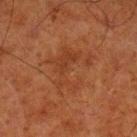<lesion>
  <image>
    <source>total-body photography crop</source>
    <field_of_view_mm>15</field_of_view_mm>
  </image>
  <site>left lower leg</site>
  <lighting>cross-polarized</lighting>
  <patient>
    <sex>male</sex>
    <age_approx>80</age_approx>
  </patient>
</lesion>